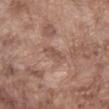Part of a total-body skin-imaging series; this lesion was reviewed on a skin check and was not flagged for biopsy.
The lesion is located on the abdomen.
A roughly 15 mm field-of-view crop from a total-body skin photograph.
A male subject about 75 years old.